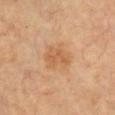biopsy status: imaged on a skin check; not biopsied | lighting: cross-polarized illumination | lesion size: ≈3.5 mm | automated metrics: roughly 7 lightness units darker than nearby skin and a normalized lesion–skin contrast near 5.5; a border-irregularity index near 2.5/10, a color-variation rating of about 3.5/10, and radial color variation of about 1; a classifier nevus-likeness of about 5/100 and a detector confidence of about 100 out of 100 that the crop contains a lesion | subject: aged around 65 | image: ~15 mm crop, total-body skin-cancer survey | body site: the arm.